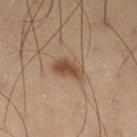Case summary:
• automated lesion analysis: an average lesion color of about L≈38 a*≈15 b*≈26 (CIELAB) and a lesion–skin lightness drop of about 9
• subject: male, aged 53 to 57
• image: ~15 mm crop, total-body skin-cancer survey
• location: the right thigh